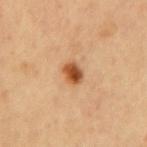Captured during whole-body skin photography for melanoma surveillance; the lesion was not biopsied.
The lesion is located on the mid back.
The lesion-visualizer software estimated an area of roughly 4 mm², an outline eccentricity of about 0.65 (0 = round, 1 = elongated), and a symmetry-axis asymmetry near 0.25. It also reported lesion-presence confidence of about 100/100.
A male patient, approximately 65 years of age.
A region of skin cropped from a whole-body photographic capture, roughly 15 mm wide.
This is a cross-polarized tile.